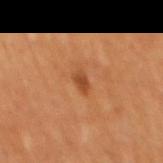Findings:
• workup: catalogued during a skin exam; not biopsied
• illumination: cross-polarized illumination
• image: ~15 mm crop, total-body skin-cancer survey
• subject: male, in their mid- to late 50s
• lesion size: ≈2.5 mm
• site: the back